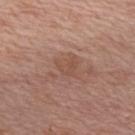  biopsy_status: not biopsied; imaged during a skin examination
  site: upper back
  lighting: white-light
  automated_metrics:
    cielab_L: 50
    cielab_a: 20
    cielab_b: 27
    vs_skin_darker_L: 6.0
    vs_skin_contrast_norm: 4.5
    border_irregularity_0_10: 5.5
    color_variation_0_10: 1.5
    peripheral_color_asymmetry: 0.5
    nevus_likeness_0_100: 0
    lesion_detection_confidence_0_100: 100
  patient:
    sex: male
    age_approx: 70
  image:
    source: total-body photography crop
    field_of_view_mm: 15